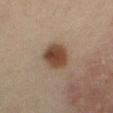Captured during whole-body skin photography for melanoma surveillance; the lesion was not biopsied. Automated image analysis of the tile measured a border-irregularity rating of about 1.5/10, a within-lesion color-variation index near 3/10, and a peripheral color-asymmetry measure near 1. Approximately 3.5 mm at its widest. The lesion is located on the leg. Imaged with cross-polarized lighting. A male patient, aged approximately 65. Cropped from a total-body skin-imaging series; the visible field is about 15 mm.